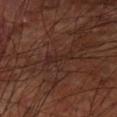biopsy status: total-body-photography surveillance lesion; no biopsy | lesion diameter: about 3 mm | illumination: cross-polarized | acquisition: ~15 mm tile from a whole-body skin photo | subject: male, about 60 years old | anatomic site: the left upper arm.